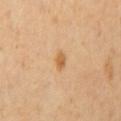Case summary:
• biopsy status — total-body-photography surveillance lesion; no biopsy
• illumination — cross-polarized illumination
• TBP lesion metrics — an area of roughly 2.5 mm², a shape eccentricity near 0.85, and a symmetry-axis asymmetry near 0.25; an average lesion color of about L≈63 a*≈21 b*≈42 (CIELAB), roughly 10 lightness units darker than nearby skin, and a lesion-to-skin contrast of about 7.5 (normalized; higher = more distinct); a lesion-detection confidence of about 100/100
• diameter — about 2.5 mm
• acquisition — ~15 mm crop, total-body skin-cancer survey
• subject — female, aged approximately 55
• anatomic site — the mid back